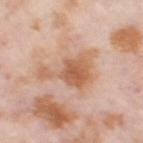patient: female, aged 53–57 | lighting: cross-polarized | size: ≈6 mm | anatomic site: the right thigh | acquisition: ~15 mm tile from a whole-body skin photo | TBP lesion metrics: a lesion area of about 15 mm², an eccentricity of roughly 0.7, and a shape-asymmetry score of about 0.6 (0 = symmetric); a border-irregularity index near 7/10, a within-lesion color-variation index near 4.5/10, and peripheral color asymmetry of about 1; a classifier nevus-likeness of about 0/100 and a detector confidence of about 100 out of 100 that the crop contains a lesion.